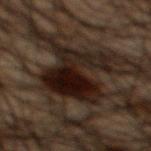– follow-up: no biopsy performed (imaged during a skin exam)
– image-analysis metrics: a peripheral color-asymmetry measure near 1.5; a nevus-likeness score of about 100/100 and a detector confidence of about 90 out of 100 that the crop contains a lesion
– body site: the mid back
– diameter: ≈7 mm
– subject: male, in their 50s
– acquisition: total-body-photography crop, ~15 mm field of view
– tile lighting: cross-polarized illumination